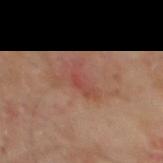The lesion was photographed on a routine skin check and not biopsied; there is no pathology result. The tile uses cross-polarized illumination. About 3.5 mm across. The patient is a male in their mid- to late 60s. A region of skin cropped from a whole-body photographic capture, roughly 15 mm wide. From the mid back.